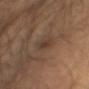notes: no biopsy performed (imaged during a skin exam)
location: the left upper arm
patient: male, aged approximately 70
acquisition: 15 mm crop, total-body photography